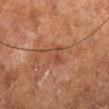This lesion was catalogued during total-body skin photography and was not selected for biopsy.
The recorded lesion diameter is about 4 mm.
A male subject about 70 years old.
A close-up tile cropped from a whole-body skin photograph, about 15 mm across.
The tile uses cross-polarized illumination.
Located on the right lower leg.